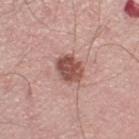{
  "biopsy_status": "not biopsied; imaged during a skin examination",
  "site": "right thigh",
  "patient": {
    "sex": "male",
    "age_approx": 70
  },
  "lighting": "white-light",
  "lesion_size": {
    "long_diameter_mm_approx": 3.5
  },
  "image": {
    "source": "total-body photography crop",
    "field_of_view_mm": 15
  }
}